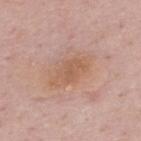This lesion was catalogued during total-body skin photography and was not selected for biopsy.
The lesion is located on the back.
Automated image analysis of the tile measured a classifier nevus-likeness of about 5/100 and a lesion-detection confidence of about 100/100.
The recorded lesion diameter is about 5.5 mm.
A roughly 15 mm field-of-view crop from a total-body skin photograph.
A male patient about 50 years old.